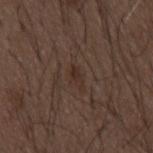Captured during whole-body skin photography for melanoma surveillance; the lesion was not biopsied. A 15 mm close-up tile from a total-body photography series done for melanoma screening. The lesion is on the mid back. The lesion's longest dimension is about 2.5 mm. The patient is a male approximately 50 years of age.